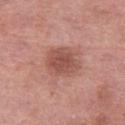| key | value |
|---|---|
| follow-up | imaged on a skin check; not biopsied |
| subject | female, about 70 years old |
| illumination | white-light illumination |
| image source | 15 mm crop, total-body photography |
| site | the left thigh |